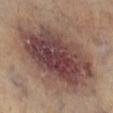Captured during whole-body skin photography for melanoma surveillance; the lesion was not biopsied.
A 15 mm crop from a total-body photograph taken for skin-cancer surveillance.
The patient is in their 60s.
The lesion is located on the leg.
Approximately 11 mm at its widest.